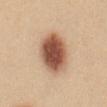Q: Lesion location?
A: the mid back
Q: Automated lesion metrics?
A: a footprint of about 18 mm², an outline eccentricity of about 0.7 (0 = round, 1 = elongated), and two-axis asymmetry of about 0.15; border irregularity of about 1.5 on a 0–10 scale, a color-variation rating of about 6.5/10, and a peripheral color-asymmetry measure near 2; an automated nevus-likeness rating near 100 out of 100
Q: What is the imaging modality?
A: ~15 mm tile from a whole-body skin photo
Q: Lesion size?
A: about 5.5 mm
Q: What are the patient's age and sex?
A: female, approximately 25 years of age
Q: Illumination type?
A: white-light illumination